Captured under cross-polarized illumination; a 15 mm close-up extracted from a 3D total-body photography capture; measured at roughly 9.5 mm in maximum diameter; the lesion is located on the upper back; a female patient aged 28 to 32; The total-body-photography lesion software estimated an area of roughly 55 mm² and a symmetry-axis asymmetry near 0.1. And it measured border irregularity of about 1 on a 0–10 scale, internal color variation of about 5 on a 0–10 scale, and radial color variation of about 1.5. It also reported a detector confidence of about 100 out of 100 that the crop contains a lesion — 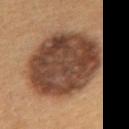Q: What is the histopathologic diagnosis?
A: a lichen planus-like keratosis (benign)